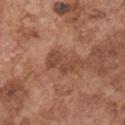{"biopsy_status": "not biopsied; imaged during a skin examination", "patient": {"sex": "male", "age_approx": 75}, "lighting": "white-light", "automated_metrics": {"area_mm2_approx": 7.5, "shape_asymmetry": 0.4, "cielab_L": 46, "cielab_a": 23, "cielab_b": 30, "border_irregularity_0_10": 5.5, "color_variation_0_10": 2.5, "peripheral_color_asymmetry": 1.0}, "site": "right upper arm", "image": {"source": "total-body photography crop", "field_of_view_mm": 15}, "lesion_size": {"long_diameter_mm_approx": 4.5}}